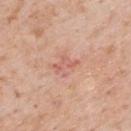• workup: total-body-photography surveillance lesion; no biopsy
• imaging modality: ~15 mm tile from a whole-body skin photo
• tile lighting: white-light illumination
• patient: male, aged 58 to 62
• location: the upper back
• lesion diameter: about 3 mm